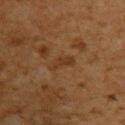This lesion was catalogued during total-body skin photography and was not selected for biopsy.
Automated image analysis of the tile measured a lesion-to-skin contrast of about 6 (normalized; higher = more distinct).
On the left upper arm.
The subject is a male in their 60s.
A roughly 15 mm field-of-view crop from a total-body skin photograph.
The recorded lesion diameter is about 3 mm.